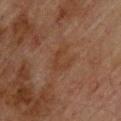Q: Is there a histopathology result?
A: total-body-photography surveillance lesion; no biopsy
Q: What is the imaging modality?
A: ~15 mm tile from a whole-body skin photo
Q: What is the anatomic site?
A: the back
Q: What are the patient's age and sex?
A: male, in their mid- to late 70s
Q: How was the tile lit?
A: cross-polarized illumination
Q: What is the lesion's diameter?
A: about 3 mm